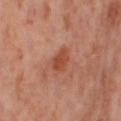Assessment:
The lesion was photographed on a routine skin check and not biopsied; there is no pathology result.
Clinical summary:
The lesion is on the right thigh. A region of skin cropped from a whole-body photographic capture, roughly 15 mm wide. The tile uses cross-polarized illumination. A female patient, aged approximately 55. Longest diameter approximately 3.5 mm.